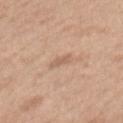Assessment: Captured during whole-body skin photography for melanoma surveillance; the lesion was not biopsied. Image and clinical context: From the mid back. A female patient, aged approximately 40. A 15 mm crop from a total-body photograph taken for skin-cancer surveillance. Longest diameter approximately 2.5 mm. The total-body-photography lesion software estimated an average lesion color of about L≈61 a*≈19 b*≈30 (CIELAB), a lesion–skin lightness drop of about 7, and a lesion-to-skin contrast of about 5 (normalized; higher = more distinct). The analysis additionally found a border-irregularity index near 3/10 and radial color variation of about 0.5. The tile uses white-light illumination.